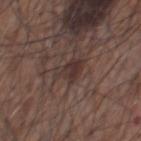Captured during whole-body skin photography for melanoma surveillance; the lesion was not biopsied. Located on the front of the torso. Measured at roughly 4 mm in maximum diameter. A close-up tile cropped from a whole-body skin photograph, about 15 mm across. A male subject, in their mid- to late 70s.